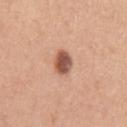Notes:
- follow-up · catalogued during a skin exam; not biopsied
- lesion diameter · ≈3 mm
- tile lighting · white-light
- automated lesion analysis · a shape-asymmetry score of about 0.1 (0 = symmetric); an average lesion color of about L≈54 a*≈24 b*≈29 (CIELAB), roughly 17 lightness units darker than nearby skin, and a normalized border contrast of about 10.5; a border-irregularity index near 1/10 and peripheral color asymmetry of about 1.5
- subject · female, aged 38–42
- image · 15 mm crop, total-body photography
- site · the left upper arm